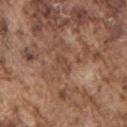Part of a total-body skin-imaging series; this lesion was reviewed on a skin check and was not flagged for biopsy. Longest diameter approximately 2.5 mm. The lesion is on the left upper arm. A roughly 15 mm field-of-view crop from a total-body skin photograph. The subject is a male approximately 75 years of age.